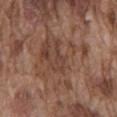Assessment:
Imaged during a routine full-body skin examination; the lesion was not biopsied and no histopathology is available.